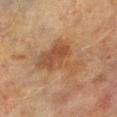{"biopsy_status": "not biopsied; imaged during a skin examination", "image": {"source": "total-body photography crop", "field_of_view_mm": 15}, "site": "right lower leg", "patient": {"sex": "male", "age_approx": 70}}